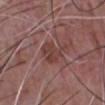acquisition=~15 mm crop, total-body skin-cancer survey; site=the chest; subject=male, about 50 years old; lighting=white-light illumination.